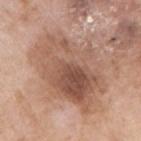The lesion was photographed on a routine skin check and not biopsied; there is no pathology result. A close-up tile cropped from a whole-body skin photograph, about 15 mm across. The subject is a female about 75 years old. The lesion is located on the right upper arm. Automated tile analysis of the lesion measured a footprint of about 41 mm², an outline eccentricity of about 0.75 (0 = round, 1 = elongated), and two-axis asymmetry of about 0.15. The analysis additionally found lesion-presence confidence of about 100/100.